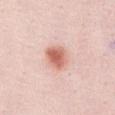This lesion was catalogued during total-body skin photography and was not selected for biopsy. About 3.5 mm across. A female subject, about 45 years old. From the abdomen. Cropped from a total-body skin-imaging series; the visible field is about 15 mm.